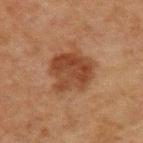Q: Was a biopsy performed?
A: no biopsy performed (imaged during a skin exam)
Q: What is the imaging modality?
A: total-body-photography crop, ~15 mm field of view
Q: What is the anatomic site?
A: the upper back
Q: What did automated image analysis measure?
A: a lesion color around L≈32 a*≈18 b*≈26 in CIELAB and roughly 9 lightness units darker than nearby skin; a classifier nevus-likeness of about 70/100 and a detector confidence of about 100 out of 100 that the crop contains a lesion
Q: What lighting was used for the tile?
A: cross-polarized
Q: Who is the patient?
A: male, approximately 60 years of age
Q: Lesion size?
A: about 5 mm